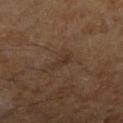workup: catalogued during a skin exam; not biopsied | anatomic site: the left lower leg | patient: male, approximately 60 years of age | image-analysis metrics: a footprint of about 3 mm² and an outline eccentricity of about 0.9 (0 = round, 1 = elongated); a mean CIELAB color near L≈28 a*≈14 b*≈22, a lesion–skin lightness drop of about 5, and a normalized border contrast of about 5.5; a within-lesion color-variation index near 0.5/10 and peripheral color asymmetry of about 0 | image source: total-body-photography crop, ~15 mm field of view | size: ≈2.5 mm | tile lighting: cross-polarized.